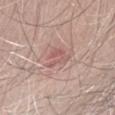<case>
<biopsy_status>not biopsied; imaged during a skin examination</biopsy_status>
</case>